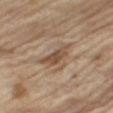Assessment:
The lesion was photographed on a routine skin check and not biopsied; there is no pathology result.
Background:
A 15 mm close-up tile from a total-body photography series done for melanoma screening. On the right thigh. A male subject about 70 years old.